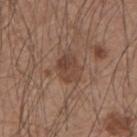Impression:
The lesion was tiled from a total-body skin photograph and was not biopsied.
Acquisition and patient details:
A male subject, approximately 55 years of age. This image is a 15 mm lesion crop taken from a total-body photograph. From the left forearm. The lesion's longest dimension is about 3 mm. The tile uses white-light illumination.